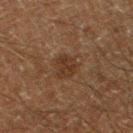workup=total-body-photography surveillance lesion; no biopsy
subject=male, about 60 years old
body site=the left thigh
image source=~15 mm tile from a whole-body skin photo